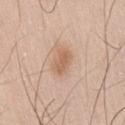This lesion was catalogued during total-body skin photography and was not selected for biopsy. An algorithmic analysis of the crop reported a border-irregularity index near 2/10, internal color variation of about 2 on a 0–10 scale, and peripheral color asymmetry of about 1. This is a white-light tile. Longest diameter approximately 3 mm. A male subject aged 58–62. Located on the right thigh. A roughly 15 mm field-of-view crop from a total-body skin photograph.